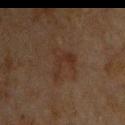Recorded during total-body skin imaging; not selected for excision or biopsy. The lesion is on the back. A 15 mm crop from a total-body photograph taken for skin-cancer surveillance. Imaged with cross-polarized lighting. Approximately 4 mm at its widest. A male subject approximately 65 years of age.